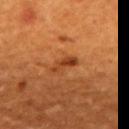Q: How large is the lesion?
A: about 3.5 mm
Q: How was the tile lit?
A: cross-polarized illumination
Q: Lesion location?
A: the back
Q: Who is the patient?
A: male, aged 58–62
Q: What kind of image is this?
A: ~15 mm crop, total-body skin-cancer survey